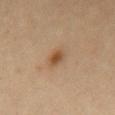notes — no biopsy performed (imaged during a skin exam) | acquisition — total-body-photography crop, ~15 mm field of view | lesion size — ≈2.5 mm | subject — male, aged 53–57 | location — the chest | tile lighting — cross-polarized illumination.